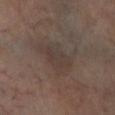Impression: Imaged during a routine full-body skin examination; the lesion was not biopsied and no histopathology is available.